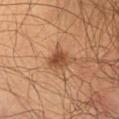notes = no biopsy performed (imaged during a skin exam)
tile lighting = cross-polarized
imaging modality = 15 mm crop, total-body photography
patient = male, about 45 years old
location = the left upper arm
TBP lesion metrics = an area of roughly 5.5 mm², a shape eccentricity near 0.5, and a symmetry-axis asymmetry near 0.25
lesion size = ~2.5 mm (longest diameter)